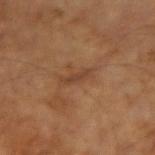Assessment:
The lesion was photographed on a routine skin check and not biopsied; there is no pathology result.
Acquisition and patient details:
The lesion is located on the left arm. A lesion tile, about 15 mm wide, cut from a 3D total-body photograph. A male patient, in their mid-60s.